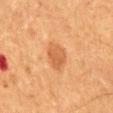Imaged during a routine full-body skin examination; the lesion was not biopsied and no histopathology is available.